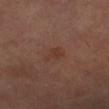Part of a total-body skin-imaging series; this lesion was reviewed on a skin check and was not flagged for biopsy.